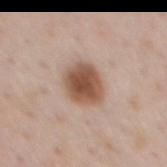| field | value |
|---|---|
| follow-up | no biopsy performed (imaged during a skin exam) |
| patient | male, about 50 years old |
| imaging modality | ~15 mm tile from a whole-body skin photo |
| location | the mid back |
| TBP lesion metrics | a classifier nevus-likeness of about 100/100 and lesion-presence confidence of about 100/100 |